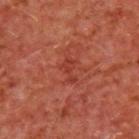Clinical summary: A 15 mm close-up tile from a total-body photography series done for melanoma screening. On the upper back. A male patient approximately 65 years of age. The recorded lesion diameter is about 3 mm.